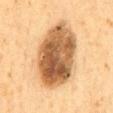– workup — catalogued during a skin exam; not biopsied
– lesion diameter — ~10 mm (longest diameter)
– tile lighting — cross-polarized illumination
– acquisition — ~15 mm crop, total-body skin-cancer survey
– site — the mid back
– subject — male, aged 58 to 62